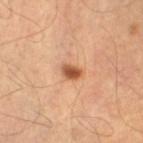Clinical impression: The lesion was photographed on a routine skin check and not biopsied; there is no pathology result. Clinical summary: Captured under cross-polarized illumination. The lesion is on the left thigh. A male patient in their 40s. Automated tile analysis of the lesion measured a lesion area of about 4 mm², an eccentricity of roughly 0.7, and a symmetry-axis asymmetry near 0.25. The software also gave an automated nevus-likeness rating near 95 out of 100 and a detector confidence of about 100 out of 100 that the crop contains a lesion. A 15 mm close-up extracted from a 3D total-body photography capture. The lesion's longest dimension is about 2.5 mm.